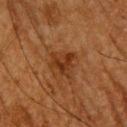| key | value |
|---|---|
| notes | no biopsy performed (imaged during a skin exam) |
| size | ~3 mm (longest diameter) |
| automated lesion analysis | an area of roughly 6 mm², an outline eccentricity of about 0.45 (0 = round, 1 = elongated), and a symmetry-axis asymmetry near 0.45; a color-variation rating of about 3/10 and a peripheral color-asymmetry measure near 1; a classifier nevus-likeness of about 10/100 and lesion-presence confidence of about 100/100 |
| subject | male, aged approximately 65 |
| image | 15 mm crop, total-body photography |
| body site | the head or neck |
| tile lighting | cross-polarized illumination |